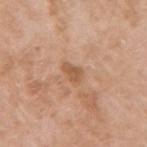Imaged with white-light lighting. A female subject, roughly 75 years of age. A roughly 15 mm field-of-view crop from a total-body skin photograph. Measured at roughly 2.5 mm in maximum diameter. The lesion is on the arm.